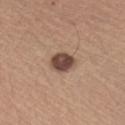Imaged during a routine full-body skin examination; the lesion was not biopsied and no histopathology is available.
From the left upper arm.
Cropped from a total-body skin-imaging series; the visible field is about 15 mm.
Longest diameter approximately 3 mm.
Captured under white-light illumination.
An algorithmic analysis of the crop reported a lesion area of about 6.5 mm², an eccentricity of roughly 0.35, and two-axis asymmetry of about 0.2. And it measured an automated nevus-likeness rating near 30 out of 100 and lesion-presence confidence of about 100/100.
The patient is a female aged 63–67.